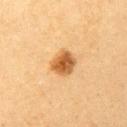Part of a total-body skin-imaging series; this lesion was reviewed on a skin check and was not flagged for biopsy.
The subject is a female aged around 20.
The total-body-photography lesion software estimated an area of roughly 7.5 mm² and a shape eccentricity near 0.45. And it measured a border-irregularity rating of about 1/10, a within-lesion color-variation index near 5/10, and a peripheral color-asymmetry measure near 1.5.
The lesion is located on the left upper arm.
Longest diameter approximately 3 mm.
A region of skin cropped from a whole-body photographic capture, roughly 15 mm wide.
This is a cross-polarized tile.